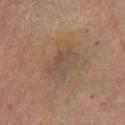Captured during whole-body skin photography for melanoma surveillance; the lesion was not biopsied. A 15 mm close-up extracted from a 3D total-body photography capture. An algorithmic analysis of the crop reported a color-variation rating of about 4.5/10 and peripheral color asymmetry of about 1.5. The lesion is on the front of the torso. Captured under white-light illumination. A male subject in their mid- to late 60s.